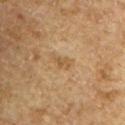The subject is a male roughly 65 years of age. The tile uses cross-polarized illumination. The lesion is on the left upper arm. A lesion tile, about 15 mm wide, cut from a 3D total-body photograph. The lesion's longest dimension is about 2.5 mm.